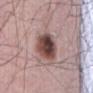A male patient, aged around 65.
This is a white-light tile.
The lesion is on the front of the torso.
An algorithmic analysis of the crop reported a footprint of about 13 mm², an outline eccentricity of about 0.6 (0 = round, 1 = elongated), and two-axis asymmetry of about 0.15. It also reported about 17 CIELAB-L* units darker than the surrounding skin and a normalized lesion–skin contrast near 12. And it measured a border-irregularity rating of about 1.5/10 and peripheral color asymmetry of about 2. The software also gave a nevus-likeness score of about 85/100 and lesion-presence confidence of about 100/100.
A 15 mm crop from a total-body photograph taken for skin-cancer surveillance.